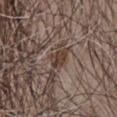Case summary:
* biopsy status — imaged on a skin check; not biopsied